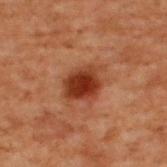{"biopsy_status": "not biopsied; imaged during a skin examination", "patient": {"sex": "male", "age_approx": 70}, "automated_metrics": {"border_irregularity_0_10": 2.5, "color_variation_0_10": 4.0, "peripheral_color_asymmetry": 1.0, "nevus_likeness_0_100": 100}, "lighting": "cross-polarized", "site": "upper back", "lesion_size": {"long_diameter_mm_approx": 4.5}, "image": {"source": "total-body photography crop", "field_of_view_mm": 15}}